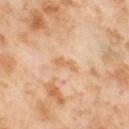  biopsy_status: not biopsied; imaged during a skin examination
  lesion_size:
    long_diameter_mm_approx: 2.5
  site: leg
  image:
    source: total-body photography crop
    field_of_view_mm: 15
  automated_metrics:
    border_irregularity_0_10: 5.0
    color_variation_0_10: 0.0
    peripheral_color_asymmetry: 0.0
  lighting: cross-polarized
  patient:
    sex: female
    age_approx: 55